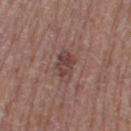biopsy status: no biopsy performed (imaged during a skin exam)
illumination: white-light
image: total-body-photography crop, ~15 mm field of view
lesion size: ~4 mm (longest diameter)
anatomic site: the left thigh
patient: female, aged 48–52
automated metrics: a border-irregularity index near 2.5/10, a color-variation rating of about 4.5/10, and peripheral color asymmetry of about 1.5; lesion-presence confidence of about 100/100